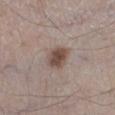  biopsy_status: not biopsied; imaged during a skin examination
  lesion_size:
    long_diameter_mm_approx: 3.0
  image:
    source: total-body photography crop
    field_of_view_mm: 15
  patient:
    sex: male
    age_approx: 60
  lighting: white-light
  site: leg